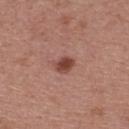follow-up = imaged on a skin check; not biopsied